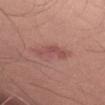{
  "biopsy_status": "not biopsied; imaged during a skin examination",
  "automated_metrics": {
    "area_mm2_approx": 5.0,
    "eccentricity": 0.9,
    "cielab_L": 50,
    "cielab_a": 26,
    "cielab_b": 24,
    "vs_skin_darker_L": 8.0,
    "vs_skin_contrast_norm": 5.5,
    "border_irregularity_0_10": 6.0,
    "color_variation_0_10": 2.5
  },
  "site": "left thigh",
  "image": {
    "source": "total-body photography crop",
    "field_of_view_mm": 15
  },
  "lighting": "white-light",
  "patient": {
    "sex": "male",
    "age_approx": 50
  }
}